From the mid back. A male patient about 40 years old. A 15 mm crop from a total-body photograph taken for skin-cancer surveillance: 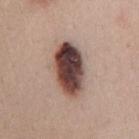Notes:
• lighting — white-light illumination
• size — ≈6.5 mm
• pathology — a dysplastic (Clark) nevus